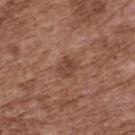follow-up: imaged on a skin check; not biopsied
patient: male, roughly 75 years of age
image-analysis metrics: a lesion area of about 4.5 mm², a shape eccentricity near 0.45, and a shape-asymmetry score of about 0.2 (0 = symmetric); a border-irregularity rating of about 2/10 and peripheral color asymmetry of about 1
lesion size: ~2.5 mm (longest diameter)
lighting: white-light illumination
body site: the upper back
acquisition: ~15 mm crop, total-body skin-cancer survey